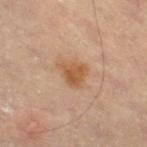The lesion is on the right thigh. Imaged with cross-polarized lighting. A male patient about 85 years old or older. Approximately 3.5 mm at its widest. Automated tile analysis of the lesion measured a lesion color around L≈53 a*≈20 b*≈34 in CIELAB, roughly 9 lightness units darker than nearby skin, and a normalized border contrast of about 7.5. A close-up tile cropped from a whole-body skin photograph, about 15 mm across.